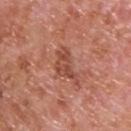  biopsy_status: not biopsied; imaged during a skin examination
  patient:
    sex: male
    age_approx: 65
  site: upper back
  lighting: white-light
  image:
    source: total-body photography crop
    field_of_view_mm: 15